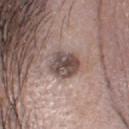- follow-up — imaged on a skin check; not biopsied
- patient — female, aged around 50
- anatomic site — the head or neck
- image source — ~15 mm crop, total-body skin-cancer survey
- diameter — about 4 mm
- tile lighting — white-light